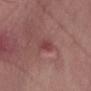  patient:
    sex: male
    age_approx: 55
  image:
    source: total-body photography crop
    field_of_view_mm: 15
  automated_metrics:
    area_mm2_approx: 3.5
    eccentricity: 0.85
    shape_asymmetry: 0.3
  site: abdomen
  lesion_size:
    long_diameter_mm_approx: 2.5
  lighting: white-light